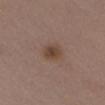tile lighting — white-light
acquisition — 15 mm crop, total-body photography
automated lesion analysis — a lesion color around L≈43 a*≈17 b*≈26 in CIELAB and a lesion–skin lightness drop of about 8; a border-irregularity index near 1.5/10; a nevus-likeness score of about 85/100 and a lesion-detection confidence of about 100/100
lesion diameter — about 2.5 mm
site — the chest
patient — male, aged approximately 40